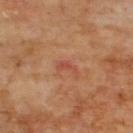Case summary:
• follow-up · imaged on a skin check; not biopsied
• anatomic site · the upper back
• patient · male, about 70 years old
• automated lesion analysis · a lesion color around L≈48 a*≈27 b*≈32 in CIELAB, a lesion–skin lightness drop of about 7, and a normalized lesion–skin contrast near 5; a border-irregularity rating of about 5.5/10, a color-variation rating of about 0/10, and peripheral color asymmetry of about 0
• illumination · cross-polarized illumination
• image source · total-body-photography crop, ~15 mm field of view
• diameter · ≈3 mm